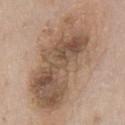{
  "lighting": "white-light",
  "image": {
    "source": "total-body photography crop",
    "field_of_view_mm": 15
  },
  "lesion_size": {
    "long_diameter_mm_approx": 13.0
  },
  "automated_metrics": {
    "cielab_L": 55,
    "cielab_a": 16,
    "cielab_b": 29,
    "vs_skin_darker_L": 12.0,
    "vs_skin_contrast_norm": 8.5,
    "border_irregularity_0_10": 4.0,
    "peripheral_color_asymmetry": 3.5
  },
  "site": "chest",
  "patient": {
    "sex": "male",
    "age_approx": 60
  }
}